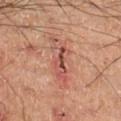Q: Was this lesion biopsied?
A: imaged on a skin check; not biopsied